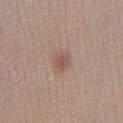Assessment:
No biopsy was performed on this lesion — it was imaged during a full skin examination and was not determined to be concerning.
Background:
Imaged with white-light lighting. On the right lower leg. The subject is a female roughly 25 years of age. A roughly 15 mm field-of-view crop from a total-body skin photograph.